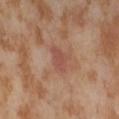A female subject, aged around 55. This is a cross-polarized tile. A roughly 15 mm field-of-view crop from a total-body skin photograph. Automated image analysis of the tile measured a footprint of about 4.5 mm², an eccentricity of roughly 0.75, and two-axis asymmetry of about 0.35. And it measured a lesion color around L≈50 a*≈24 b*≈28 in CIELAB. The analysis additionally found a classifier nevus-likeness of about 0/100 and a detector confidence of about 100 out of 100 that the crop contains a lesion. From the abdomen. Longest diameter approximately 3 mm.